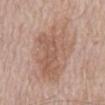Case summary:
- follow-up · total-body-photography surveillance lesion; no biopsy
- site · the abdomen
- subject · male, roughly 70 years of age
- image · total-body-photography crop, ~15 mm field of view
- size · ≈10 mm
- illumination · white-light